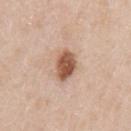Recorded during total-body skin imaging; not selected for excision or biopsy. The lesion's longest dimension is about 3.5 mm. Cropped from a total-body skin-imaging series; the visible field is about 15 mm. Captured under white-light illumination. A male subject aged 48 to 52. The lesion is located on the arm.